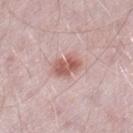Q: What kind of image is this?
A: ~15 mm crop, total-body skin-cancer survey
Q: What is the lesion's diameter?
A: ~3.5 mm (longest diameter)
Q: Lesion location?
A: the left thigh
Q: What did automated image analysis measure?
A: a footprint of about 7 mm², a shape eccentricity near 0.6, and a shape-asymmetry score of about 0.2 (0 = symmetric); a mean CIELAB color near L≈58 a*≈23 b*≈24, roughly 12 lightness units darker than nearby skin, and a normalized border contrast of about 8.5; a border-irregularity rating of about 2.5/10, internal color variation of about 3 on a 0–10 scale, and a peripheral color-asymmetry measure near 1; a lesion-detection confidence of about 100/100
Q: Illumination type?
A: white-light
Q: Who is the patient?
A: male, approximately 50 years of age